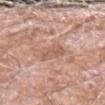follow-up = catalogued during a skin exam; not biopsied
site = the left forearm
patient = male, in their 60s
imaging modality = ~15 mm tile from a whole-body skin photo